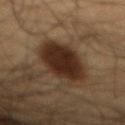{
  "biopsy_status": "not biopsied; imaged during a skin examination",
  "automated_metrics": {
    "eccentricity": 0.9,
    "shape_asymmetry": 0.15,
    "cielab_L": 25,
    "cielab_a": 15,
    "cielab_b": 23,
    "vs_skin_darker_L": 14.0,
    "border_irregularity_0_10": 2.0,
    "color_variation_0_10": 3.0,
    "peripheral_color_asymmetry": 1.0,
    "nevus_likeness_0_100": 100,
    "lesion_detection_confidence_0_100": 100
  },
  "lighting": "cross-polarized",
  "image": {
    "source": "total-body photography crop",
    "field_of_view_mm": 15
  },
  "patient": {
    "sex": "male",
    "age_approx": 60
  },
  "site": "abdomen"
}